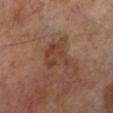No biopsy was performed on this lesion — it was imaged during a full skin examination and was not determined to be concerning.
Automated image analysis of the tile measured a footprint of about 8 mm² and a shape eccentricity near 0.65. The software also gave a lesion-detection confidence of about 100/100.
The recorded lesion diameter is about 4.5 mm.
The subject is a male aged approximately 70.
Imaged with cross-polarized lighting.
The lesion is located on the left lower leg.
A region of skin cropped from a whole-body photographic capture, roughly 15 mm wide.